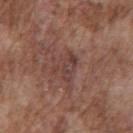biopsy_status: not biopsied; imaged during a skin examination
patient:
  sex: male
  age_approx: 75
site: chest
image:
  source: total-body photography crop
  field_of_view_mm: 15